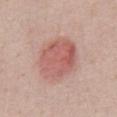Assessment: Part of a total-body skin-imaging series; this lesion was reviewed on a skin check and was not flagged for biopsy. Image and clinical context: Longest diameter approximately 5.5 mm. On the abdomen. A lesion tile, about 15 mm wide, cut from a 3D total-body photograph. This is a white-light tile. A male subject in their 40s.